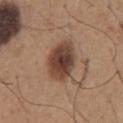Imaged during a routine full-body skin examination; the lesion was not biopsied and no histopathology is available. From the chest. A 15 mm crop from a total-body photograph taken for skin-cancer surveillance. The subject is a male aged approximately 65. This is a white-light tile. The lesion's longest dimension is about 5.5 mm.